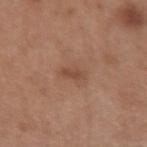workup = no biopsy performed (imaged during a skin exam)
body site = the right forearm
patient = female, aged approximately 30
lesion diameter = ≈3 mm
tile lighting = white-light
image = 15 mm crop, total-body photography